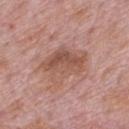site: the mid back | patient: male, aged around 75 | illumination: white-light | image: 15 mm crop, total-body photography.